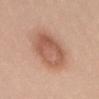Image and clinical context:
The recorded lesion diameter is about 6.5 mm. Cropped from a whole-body photographic skin survey; the tile spans about 15 mm. The subject is a female roughly 30 years of age. The lesion is located on the abdomen. This is a white-light tile. Automated image analysis of the tile measured a lesion area of about 21 mm², an outline eccentricity of about 0.75 (0 = round, 1 = elongated), and a shape-asymmetry score of about 0.1 (0 = symmetric). The analysis additionally found border irregularity of about 1.5 on a 0–10 scale, a color-variation rating of about 5/10, and peripheral color asymmetry of about 1.5.